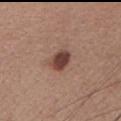Q: Is there a histopathology result?
A: imaged on a skin check; not biopsied
Q: Lesion size?
A: ≈3 mm
Q: How was this image acquired?
A: ~15 mm crop, total-body skin-cancer survey
Q: Who is the patient?
A: female, about 65 years old
Q: Illumination type?
A: white-light illumination
Q: What is the anatomic site?
A: the chest